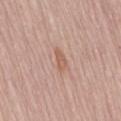Assessment: This lesion was catalogued during total-body skin photography and was not selected for biopsy. Clinical summary: A female patient, approximately 65 years of age. The lesion is located on the lower back. The tile uses white-light illumination. Cropped from a total-body skin-imaging series; the visible field is about 15 mm.